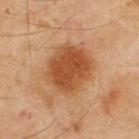Q: Was this lesion biopsied?
A: imaged on a skin check; not biopsied
Q: Patient demographics?
A: male, aged around 45
Q: Lesion location?
A: the back
Q: What is the lesion's diameter?
A: ≈5.5 mm
Q: Automated lesion metrics?
A: a lesion area of about 23 mm² and an outline eccentricity of about 0.5 (0 = round, 1 = elongated); a border-irregularity index near 1.5/10, a within-lesion color-variation index near 3.5/10, and a peripheral color-asymmetry measure near 1; a nevus-likeness score of about 90/100 and lesion-presence confidence of about 100/100
Q: What kind of image is this?
A: ~15 mm crop, total-body skin-cancer survey